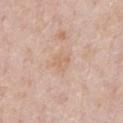This lesion was catalogued during total-body skin photography and was not selected for biopsy. A 15 mm close-up tile from a total-body photography series done for melanoma screening. From the front of the torso. The subject is a male aged around 50. Captured under white-light illumination.